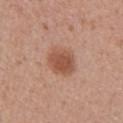No biopsy was performed on this lesion — it was imaged during a full skin examination and was not determined to be concerning.
The lesion is located on the left upper arm.
About 4 mm across.
The lesion-visualizer software estimated roughly 11 lightness units darker than nearby skin and a normalized lesion–skin contrast near 8. It also reported a border-irregularity rating of about 1.5/10, a color-variation rating of about 3/10, and a peripheral color-asymmetry measure near 1.
The tile uses white-light illumination.
A 15 mm crop from a total-body photograph taken for skin-cancer surveillance.
A female patient, about 60 years old.